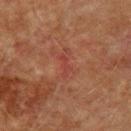| field | value |
|---|---|
| notes | imaged on a skin check; not biopsied |
| acquisition | 15 mm crop, total-body photography |
| patient | male, in their mid- to late 70s |
| anatomic site | the arm |